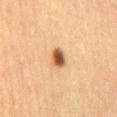notes = total-body-photography surveillance lesion; no biopsy
lighting = cross-polarized
body site = the mid back
image = ~15 mm crop, total-body skin-cancer survey
diameter = about 2.5 mm
patient = female, roughly 60 years of age